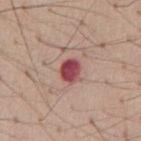workup: total-body-photography surveillance lesion; no biopsy
site: the abdomen
image source: ~15 mm crop, total-body skin-cancer survey
subject: male, aged approximately 55
tile lighting: white-light